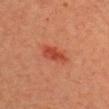No biopsy was performed on this lesion — it was imaged during a full skin examination and was not determined to be concerning.
Measured at roughly 3.5 mm in maximum diameter.
On the head or neck.
A male patient aged 38 to 42.
Cropped from a total-body skin-imaging series; the visible field is about 15 mm.
This is a cross-polarized tile.